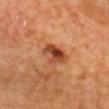Context: Imaged with cross-polarized lighting. Cropped from a total-body skin-imaging series; the visible field is about 15 mm. Longest diameter approximately 3.5 mm. From the chest. The subject is a male aged 68 to 72.